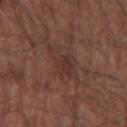Clinical impression: The lesion was tiled from a total-body skin photograph and was not biopsied. Context: The lesion is located on the right forearm. The recorded lesion diameter is about 5.5 mm. A region of skin cropped from a whole-body photographic capture, roughly 15 mm wide. Imaged with white-light lighting. The subject is a male in their mid- to late 60s. An algorithmic analysis of the crop reported peripheral color asymmetry of about 1. And it measured a lesion-detection confidence of about 65/100.